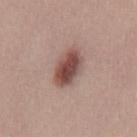{"biopsy_status": "not biopsied; imaged during a skin examination", "lesion_size": {"long_diameter_mm_approx": 5.0}, "patient": {"sex": "male", "age_approx": 30}, "automated_metrics": {"cielab_L": 48, "cielab_a": 21, "cielab_b": 23, "vs_skin_contrast_norm": 10.5, "border_irregularity_0_10": 2.0, "color_variation_0_10": 4.5, "peripheral_color_asymmetry": 1.5, "nevus_likeness_0_100": 95, "lesion_detection_confidence_0_100": 100}, "image": {"source": "total-body photography crop", "field_of_view_mm": 15}, "lighting": "white-light"}